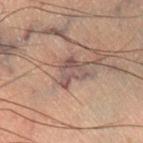Assessment:
No biopsy was performed on this lesion — it was imaged during a full skin examination and was not determined to be concerning.
Background:
The lesion is located on the right lower leg. The subject is a male about 50 years old. This is a cross-polarized tile. The lesion's longest dimension is about 3.5 mm. A region of skin cropped from a whole-body photographic capture, roughly 15 mm wide.